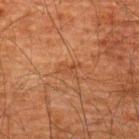  biopsy_status: not biopsied; imaged during a skin examination
  automated_metrics:
    area_mm2_approx: 3.0
    eccentricity: 0.85
    shape_asymmetry: 0.45
    cielab_L: 37
    cielab_a: 20
    cielab_b: 30
    vs_skin_contrast_norm: 4.5
    border_irregularity_0_10: 5.5
    color_variation_0_10: 0.0
    peripheral_color_asymmetry: 0.0
    nevus_likeness_0_100: 0
  image:
    source: total-body photography crop
    field_of_view_mm: 15
  lighting: cross-polarized
  lesion_size:
    long_diameter_mm_approx: 3.0
  patient:
    sex: male
    age_approx: 60
  site: upper back